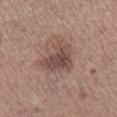biopsy_status: not biopsied; imaged during a skin examination
site: right lower leg
patient:
  sex: female
  age_approx: 65
automated_metrics:
  area_mm2_approx: 11.0
  eccentricity: 0.6
  shape_asymmetry: 0.45
  cielab_L: 47
  cielab_a: 18
  cielab_b: 23
  vs_skin_darker_L: 10.0
  vs_skin_contrast_norm: 7.5
  border_irregularity_0_10: 4.5
  color_variation_0_10: 3.5
  peripheral_color_asymmetry: 1.0
  nevus_likeness_0_100: 15
image:
  source: total-body photography crop
  field_of_view_mm: 15
lesion_size:
  long_diameter_mm_approx: 4.0